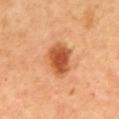Q: Was a biopsy performed?
A: total-body-photography surveillance lesion; no biopsy
Q: Who is the patient?
A: male, aged around 65
Q: Where on the body is the lesion?
A: the back
Q: What kind of image is this?
A: ~15 mm crop, total-body skin-cancer survey